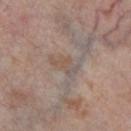Part of a total-body skin-imaging series; this lesion was reviewed on a skin check and was not flagged for biopsy. On the left thigh. A female subject aged 53 to 57. Approximately 3.5 mm at its widest. This is a cross-polarized tile. A lesion tile, about 15 mm wide, cut from a 3D total-body photograph.